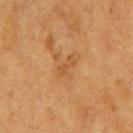follow-up: no biopsy performed (imaged during a skin exam)
acquisition: ~15 mm crop, total-body skin-cancer survey
subject: female, in their mid- to late 50s
tile lighting: cross-polarized illumination
diameter: ≈3 mm
site: the arm